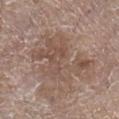Assessment:
This lesion was catalogued during total-body skin photography and was not selected for biopsy.
Acquisition and patient details:
A close-up tile cropped from a whole-body skin photograph, about 15 mm across. Longest diameter approximately 7.5 mm. The lesion-visualizer software estimated an outline eccentricity of about 0.75 (0 = round, 1 = elongated). And it measured an average lesion color of about L≈50 a*≈16 b*≈24 (CIELAB), a lesion–skin lightness drop of about 8, and a normalized border contrast of about 6. A male patient about 65 years old. Imaged with white-light lighting. The lesion is located on the right lower leg.